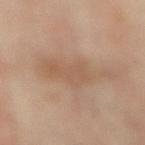Q: Is there a histopathology result?
A: no biopsy performed (imaged during a skin exam)
Q: What is the imaging modality?
A: total-body-photography crop, ~15 mm field of view
Q: Automated lesion metrics?
A: a lesion area of about 15 mm² and a shape-asymmetry score of about 0.4 (0 = symmetric); about 6 CIELAB-L* units darker than the surrounding skin and a lesion-to-skin contrast of about 5 (normalized; higher = more distinct); lesion-presence confidence of about 100/100
Q: Where on the body is the lesion?
A: the front of the torso
Q: What is the lesion's diameter?
A: about 6.5 mm
Q: What lighting was used for the tile?
A: cross-polarized illumination
Q: Patient demographics?
A: female, in their mid- to late 60s